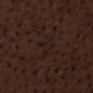| key | value |
|---|---|
| biopsy status | catalogued during a skin exam; not biopsied |
| automated metrics | border irregularity of about 5.5 on a 0–10 scale, a within-lesion color-variation index near 1.5/10, and peripheral color asymmetry of about 0.5 |
| body site | the chest |
| size | ~3 mm (longest diameter) |
| acquisition | 15 mm crop, total-body photography |
| patient | male, in their 70s |